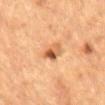{
  "biopsy_status": "not biopsied; imaged during a skin examination"
}